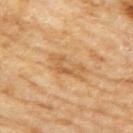{"biopsy_status": "not biopsied; imaged during a skin examination", "patient": {"sex": "female", "age_approx": 60}, "lighting": "cross-polarized", "site": "right upper arm", "automated_metrics": {"area_mm2_approx": 6.0, "eccentricity": 0.85, "shape_asymmetry": 0.6, "cielab_L": 60, "cielab_a": 21, "cielab_b": 41, "vs_skin_darker_L": 8.0, "vs_skin_contrast_norm": 5.5, "border_irregularity_0_10": 7.5, "color_variation_0_10": 2.5, "peripheral_color_asymmetry": 0.5}, "lesion_size": {"long_diameter_mm_approx": 4.0}, "image": {"source": "total-body photography crop", "field_of_view_mm": 15}}